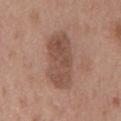Imaged during a routine full-body skin examination; the lesion was not biopsied and no histopathology is available.
The lesion is on the chest.
This is a white-light tile.
A close-up tile cropped from a whole-body skin photograph, about 15 mm across.
About 7.5 mm across.
A male subject in their mid- to late 50s.